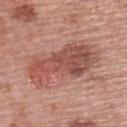| field | value |
|---|---|
| biopsy status | imaged on a skin check; not biopsied |
| diameter | ~9 mm (longest diameter) |
| image source | total-body-photography crop, ~15 mm field of view |
| site | the back |
| subject | female, approximately 60 years of age |